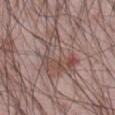| field | value |
|---|---|
| notes | total-body-photography surveillance lesion; no biopsy |
| acquisition | total-body-photography crop, ~15 mm field of view |
| anatomic site | the abdomen |
| subject | male, about 65 years old |